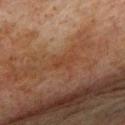No biopsy was performed on this lesion — it was imaged during a full skin examination and was not determined to be concerning.
A roughly 15 mm field-of-view crop from a total-body skin photograph.
From the upper back.
The patient is a male aged around 75.
Measured at roughly 2.5 mm in maximum diameter.